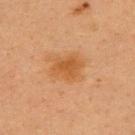Q: Was a biopsy performed?
A: imaged on a skin check; not biopsied
Q: Automated lesion metrics?
A: an area of roughly 7.5 mm², an outline eccentricity of about 0.65 (0 = round, 1 = elongated), and two-axis asymmetry of about 0.25; a mean CIELAB color near L≈47 a*≈22 b*≈38 and roughly 8 lightness units darker than nearby skin; a color-variation rating of about 2/10 and peripheral color asymmetry of about 0.5
Q: How large is the lesion?
A: ~3.5 mm (longest diameter)
Q: What are the patient's age and sex?
A: female, roughly 40 years of age
Q: Where on the body is the lesion?
A: the upper back
Q: What lighting was used for the tile?
A: cross-polarized
Q: How was this image acquired?
A: ~15 mm tile from a whole-body skin photo